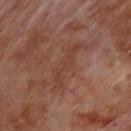Assessment:
The lesion was tiled from a total-body skin photograph and was not biopsied.
Acquisition and patient details:
Measured at roughly 6 mm in maximum diameter. A male subject, approximately 70 years of age. From the upper back. A roughly 15 mm field-of-view crop from a total-body skin photograph. This is a cross-polarized tile.